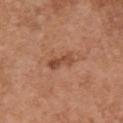workup = total-body-photography surveillance lesion; no biopsy | body site = the chest | subject = female, roughly 65 years of age | image = 15 mm crop, total-body photography | size = ~3.5 mm (longest diameter).